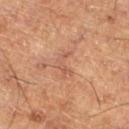<record>
<biopsy_status>not biopsied; imaged during a skin examination</biopsy_status>
<patient>
  <sex>male</sex>
  <age_approx>60</age_approx>
</patient>
<site>left lower leg</site>
<image>
  <source>total-body photography crop</source>
  <field_of_view_mm>15</field_of_view_mm>
</image>
<lesion_size>
  <long_diameter_mm_approx>3.5</long_diameter_mm_approx>
</lesion_size>
</record>